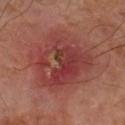follow-up = imaged on a skin check; not biopsied
subject = male, aged approximately 70
TBP lesion metrics = a lesion area of about 32 mm², an eccentricity of roughly 0.35, and two-axis asymmetry of about 0.25; a border-irregularity rating of about 3.5/10, internal color variation of about 9.5 on a 0–10 scale, and radial color variation of about 3.5; a classifier nevus-likeness of about 0/100
image source = total-body-photography crop, ~15 mm field of view
lesion size = about 6.5 mm
location = the left forearm
tile lighting = cross-polarized illumination